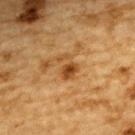Imaged during a routine full-body skin examination; the lesion was not biopsied and no histopathology is available.
The subject is a male roughly 85 years of age or older.
The total-body-photography lesion software estimated a mean CIELAB color near L≈42 a*≈21 b*≈38, roughly 10 lightness units darker than nearby skin, and a normalized border contrast of about 8. The software also gave a border-irregularity rating of about 5/10 and a color-variation rating of about 3/10. The software also gave an automated nevus-likeness rating near 60 out of 100 and a detector confidence of about 100 out of 100 that the crop contains a lesion.
A region of skin cropped from a whole-body photographic capture, roughly 15 mm wide.
Located on the upper back.
This is a cross-polarized tile.
The recorded lesion diameter is about 3 mm.